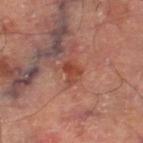Part of a total-body skin-imaging series; this lesion was reviewed on a skin check and was not flagged for biopsy.
A 15 mm close-up tile from a total-body photography series done for melanoma screening.
Imaged with cross-polarized lighting.
Longest diameter approximately 2.5 mm.
The lesion is on the right thigh.
Automated image analysis of the tile measured an area of roughly 4 mm², a shape eccentricity near 0.65, and a shape-asymmetry score of about 0.35 (0 = symmetric). The analysis additionally found a lesion–skin lightness drop of about 9 and a normalized border contrast of about 7.5. It also reported border irregularity of about 3.5 on a 0–10 scale, a color-variation rating of about 2/10, and peripheral color asymmetry of about 0.5. And it measured a nevus-likeness score of about 5/100.